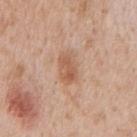follow-up = catalogued during a skin exam; not biopsied
lighting = white-light illumination
image-analysis metrics = a lesion–skin lightness drop of about 9 and a normalized border contrast of about 6.5; a border-irregularity rating of about 2/10, a within-lesion color-variation index near 3.5/10, and a peripheral color-asymmetry measure near 1; a classifier nevus-likeness of about 50/100 and lesion-presence confidence of about 100/100
site = the mid back
image source = ~15 mm tile from a whole-body skin photo
patient = male, aged around 65
size = ~3.5 mm (longest diameter)